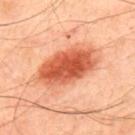Background: A 15 mm close-up extracted from a 3D total-body photography capture. The subject is a male about 50 years old. This is a cross-polarized tile. The lesion is on the back. Automated tile analysis of the lesion measured an automated nevus-likeness rating near 100 out of 100.